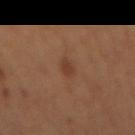{
  "biopsy_status": "not biopsied; imaged during a skin examination",
  "automated_metrics": {
    "area_mm2_approx": 2.5,
    "eccentricity": 0.9,
    "shape_asymmetry": 0.2,
    "cielab_L": 38,
    "cielab_a": 21,
    "cielab_b": 30,
    "vs_skin_darker_L": 7.0,
    "border_irregularity_0_10": 2.5,
    "peripheral_color_asymmetry": 0.0
  },
  "lighting": "cross-polarized",
  "patient": {
    "sex": "female"
  },
  "image": {
    "source": "total-body photography crop",
    "field_of_view_mm": 15
  },
  "site": "mid back",
  "lesion_size": {
    "long_diameter_mm_approx": 2.5
  }
}